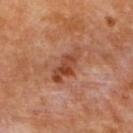Impression:
Part of a total-body skin-imaging series; this lesion was reviewed on a skin check and was not flagged for biopsy.
Image and clinical context:
A 15 mm close-up tile from a total-body photography series done for melanoma screening. The subject is a female approximately 50 years of age. Captured under cross-polarized illumination. The lesion is on the upper back. The recorded lesion diameter is about 5 mm.